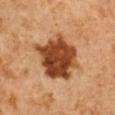workup = total-body-photography surveillance lesion; no biopsy
image = ~15 mm tile from a whole-body skin photo
size = ~5.5 mm (longest diameter)
subject = male, approximately 60 years of age
body site = the right arm
automated lesion analysis = a border-irregularity index near 3/10, a within-lesion color-variation index near 5/10, and a peripheral color-asymmetry measure near 2
lighting = cross-polarized illumination